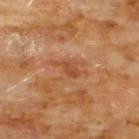The lesion was tiled from a total-body skin photograph and was not biopsied. The patient is a male aged 58–62. A 15 mm crop from a total-body photograph taken for skin-cancer surveillance. From the chest.